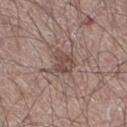Q: Was this lesion biopsied?
A: catalogued during a skin exam; not biopsied
Q: Lesion size?
A: about 3.5 mm
Q: What is the anatomic site?
A: the leg
Q: Automated lesion metrics?
A: roughly 9 lightness units darker than nearby skin and a normalized lesion–skin contrast near 7; border irregularity of about 4 on a 0–10 scale and a peripheral color-asymmetry measure near 1; a detector confidence of about 100 out of 100 that the crop contains a lesion
Q: Who is the patient?
A: male, in their mid-60s
Q: How was this image acquired?
A: ~15 mm tile from a whole-body skin photo